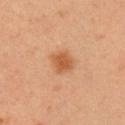Case summary:
* biopsy status · total-body-photography surveillance lesion; no biopsy